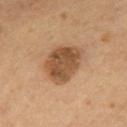workup: total-body-photography surveillance lesion; no biopsy
imaging modality: ~15 mm crop, total-body skin-cancer survey
anatomic site: the mid back
illumination: cross-polarized
automated lesion analysis: a footprint of about 15 mm², an outline eccentricity of about 0.7 (0 = round, 1 = elongated), and a shape-asymmetry score of about 0.2 (0 = symmetric); a mean CIELAB color near L≈45 a*≈18 b*≈32; border irregularity of about 2 on a 0–10 scale, internal color variation of about 3.5 on a 0–10 scale, and a peripheral color-asymmetry measure near 1; a classifier nevus-likeness of about 35/100 and a detector confidence of about 100 out of 100 that the crop contains a lesion
subject: male, in their mid- to late 50s